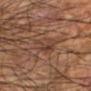Impression:
The lesion was tiled from a total-body skin photograph and was not biopsied.
Image and clinical context:
The lesion is on the left forearm. An algorithmic analysis of the crop reported a mean CIELAB color near L≈40 a*≈18 b*≈27, about 6 CIELAB-L* units darker than the surrounding skin, and a normalized border contrast of about 5. The subject is a male aged 58 to 62. A 15 mm crop from a total-body photograph taken for skin-cancer surveillance.